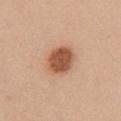A female subject, aged around 45. The total-body-photography lesion software estimated a lesion area of about 10 mm², an eccentricity of roughly 0.4, and a shape-asymmetry score of about 0.1 (0 = symmetric). The software also gave a lesion color around L≈54 a*≈24 b*≈33 in CIELAB, about 15 CIELAB-L* units darker than the surrounding skin, and a lesion-to-skin contrast of about 10 (normalized; higher = more distinct). And it measured a border-irregularity index near 1/10, a color-variation rating of about 4/10, and radial color variation of about 1.5. The lesion is located on the chest. Measured at roughly 3.5 mm in maximum diameter. A 15 mm close-up extracted from a 3D total-body photography capture.